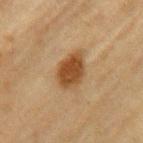Q: Is there a histopathology result?
A: no biopsy performed (imaged during a skin exam)
Q: Patient demographics?
A: male, about 70 years old
Q: Where on the body is the lesion?
A: the left upper arm
Q: What did automated image analysis measure?
A: an average lesion color of about L≈39 a*≈17 b*≈32 (CIELAB), about 11 CIELAB-L* units darker than the surrounding skin, and a normalized border contrast of about 10; a border-irregularity rating of about 2/10 and peripheral color asymmetry of about 1
Q: Lesion size?
A: ~4.5 mm (longest diameter)
Q: Illumination type?
A: cross-polarized
Q: What is the imaging modality?
A: ~15 mm crop, total-body skin-cancer survey